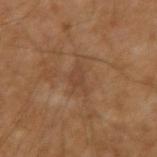follow-up — total-body-photography surveillance lesion; no biopsy | lesion diameter — about 3 mm | illumination — cross-polarized illumination | patient — male, aged 53–57 | acquisition — total-body-photography crop, ~15 mm field of view | site — the arm | TBP lesion metrics — a lesion color around L≈39 a*≈17 b*≈29 in CIELAB, a lesion–skin lightness drop of about 6, and a normalized border contrast of about 5.